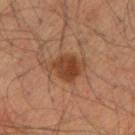The lesion was photographed on a routine skin check and not biopsied; there is no pathology result. The lesion is on the right upper arm. Cropped from a total-body skin-imaging series; the visible field is about 15 mm. The patient is a male aged around 35.